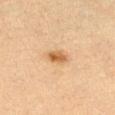Notes:
- patient: female, aged 63–67
- size: ≈2.5 mm
- image source: 15 mm crop, total-body photography
- tile lighting: cross-polarized
- site: the left thigh